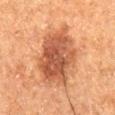Impression: This lesion was catalogued during total-body skin photography and was not selected for biopsy. Acquisition and patient details: The tile uses cross-polarized illumination. The lesion's longest dimension is about 7.5 mm. Cropped from a whole-body photographic skin survey; the tile spans about 15 mm. A male subject, aged 73–77. Located on the right thigh. The lesion-visualizer software estimated an area of roughly 28 mm², an eccentricity of roughly 0.7, and a symmetry-axis asymmetry near 0.15. The analysis additionally found an automated nevus-likeness rating near 70 out of 100 and a detector confidence of about 100 out of 100 that the crop contains a lesion.